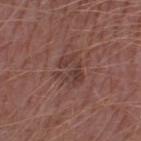Recorded during total-body skin imaging; not selected for excision or biopsy.